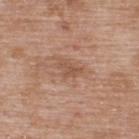Findings:
* follow-up: imaged on a skin check; not biopsied
* size: ≈3 mm
* image: ~15 mm tile from a whole-body skin photo
* tile lighting: white-light
* body site: the upper back
* image-analysis metrics: an outline eccentricity of about 0.8 (0 = round, 1 = elongated)
* subject: male, aged around 50